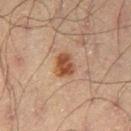Assessment: Recorded during total-body skin imaging; not selected for excision or biopsy. Acquisition and patient details: On the left thigh. The tile uses cross-polarized illumination. The total-body-photography lesion software estimated border irregularity of about 2 on a 0–10 scale and a color-variation rating of about 3.5/10. The patient is a male roughly 70 years of age. About 3 mm across. Cropped from a total-body skin-imaging series; the visible field is about 15 mm.